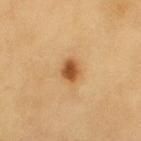<lesion>
<biopsy_status>not biopsied; imaged during a skin examination</biopsy_status>
<patient>
  <sex>male</sex>
  <age_approx>60</age_approx>
</patient>
<lighting>cross-polarized</lighting>
<image>
  <source>total-body photography crop</source>
  <field_of_view_mm>15</field_of_view_mm>
</image>
<site>left upper arm</site>
</lesion>